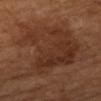Assessment:
The lesion was tiled from a total-body skin photograph and was not biopsied.